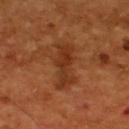Q: What lighting was used for the tile?
A: cross-polarized
Q: Lesion size?
A: about 5.5 mm
Q: Where on the body is the lesion?
A: the back
Q: What kind of image is this?
A: 15 mm crop, total-body photography
Q: What are the patient's age and sex?
A: male, in their mid- to late 50s
Q: Automated lesion metrics?
A: a footprint of about 11 mm², a shape eccentricity near 0.9, and a symmetry-axis asymmetry near 0.3; a color-variation rating of about 3.5/10 and peripheral color asymmetry of about 1.5; an automated nevus-likeness rating near 0 out of 100 and a detector confidence of about 100 out of 100 that the crop contains a lesion